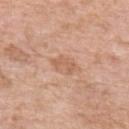follow-up = total-body-photography surveillance lesion; no biopsy | illumination = white-light illumination | anatomic site = the left upper arm | patient = female, approximately 75 years of age | acquisition = ~15 mm tile from a whole-body skin photo.